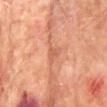<record>
  <lesion_size>
    <long_diameter_mm_approx>3.5</long_diameter_mm_approx>
  </lesion_size>
  <site>mid back</site>
  <image>
    <source>total-body photography crop</source>
    <field_of_view_mm>15</field_of_view_mm>
  </image>
  <lighting>cross-polarized</lighting>
  <patient>
    <sex>male</sex>
    <age_approx>75</age_approx>
  </patient>
</record>